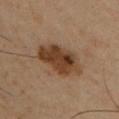Assessment:
Imaged during a routine full-body skin examination; the lesion was not biopsied and no histopathology is available.
Image and clinical context:
The recorded lesion diameter is about 5.5 mm. The total-body-photography lesion software estimated border irregularity of about 2.5 on a 0–10 scale, a color-variation rating of about 5.5/10, and peripheral color asymmetry of about 2. The lesion is located on the chest. Captured under cross-polarized illumination. A male patient, in their 50s. A 15 mm close-up tile from a total-body photography series done for melanoma screening.